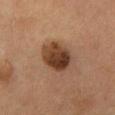Impression: The lesion was photographed on a routine skin check and not biopsied; there is no pathology result. Image and clinical context: A region of skin cropped from a whole-body photographic capture, roughly 15 mm wide. Imaged with cross-polarized lighting. Located on the chest. A male patient, aged approximately 55. About 4.5 mm across.